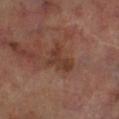automated lesion analysis: a shape eccentricity near 0.7
acquisition: total-body-photography crop, ~15 mm field of view
size: ≈4 mm
tile lighting: cross-polarized illumination
patient: male, roughly 70 years of age
site: the right lower leg